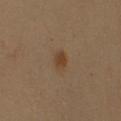  biopsy_status: not biopsied; imaged during a skin examination
  patient:
    sex: female
    age_approx: 45
  image:
    source: total-body photography crop
    field_of_view_mm: 15
  lesion_size:
    long_diameter_mm_approx: 2.5
  site: left arm